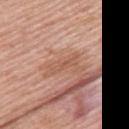size: ≈4 mm
subject: female, about 60 years old
acquisition: 15 mm crop, total-body photography
location: the upper back
automated metrics: a lesion color around L≈56 a*≈22 b*≈31 in CIELAB, a lesion–skin lightness drop of about 7, and a normalized lesion–skin contrast near 5.5; a color-variation rating of about 0/10 and a peripheral color-asymmetry measure near 0